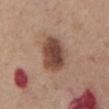Assessment:
Part of a total-body skin-imaging series; this lesion was reviewed on a skin check and was not flagged for biopsy.
Acquisition and patient details:
The tile uses white-light illumination. A 15 mm close-up tile from a total-body photography series done for melanoma screening. The lesion's longest dimension is about 4.5 mm. The patient is a male approximately 75 years of age. Located on the chest.